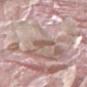Assessment: This lesion was catalogued during total-body skin photography and was not selected for biopsy. Image and clinical context: About 2.5 mm across. The patient is a male aged 38 to 42. The total-body-photography lesion software estimated a shape eccentricity near 0.7. The software also gave a lesion–skin lightness drop of about 10 and a normalized lesion–skin contrast near 7. And it measured a border-irregularity rating of about 2.5/10 and a peripheral color-asymmetry measure near 0.5. Cropped from a total-body skin-imaging series; the visible field is about 15 mm. The lesion is on the left thigh.